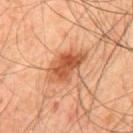biopsy status: imaged on a skin check; not biopsied.